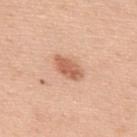Imaged during a routine full-body skin examination; the lesion was not biopsied and no histopathology is available. The tile uses white-light illumination. The lesion is on the upper back. The patient is a male aged around 50. A 15 mm close-up extracted from a 3D total-body photography capture.